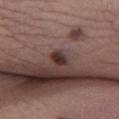Part of a total-body skin-imaging series; this lesion was reviewed on a skin check and was not flagged for biopsy. The subject is a male roughly 40 years of age. The tile uses white-light illumination. Approximately 2.5 mm at its widest. A roughly 15 mm field-of-view crop from a total-body skin photograph. An algorithmic analysis of the crop reported a lesion color around L≈31 a*≈18 b*≈18 in CIELAB and a normalized lesion–skin contrast near 11.5. It also reported border irregularity of about 2.5 on a 0–10 scale, a color-variation rating of about 2/10, and radial color variation of about 0.5. The software also gave a classifier nevus-likeness of about 90/100 and lesion-presence confidence of about 100/100. The lesion is on the leg.